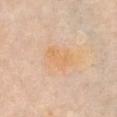Assessment:
Recorded during total-body skin imaging; not selected for excision or biopsy.
Background:
The patient is a female in their mid-60s. From the chest. This is a white-light tile. Cropped from a total-body skin-imaging series; the visible field is about 15 mm.